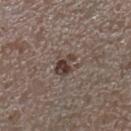follow-up: total-body-photography surveillance lesion; no biopsy
subject: female, about 70 years old
size: about 3.5 mm
lighting: white-light
anatomic site: the right lower leg
image: 15 mm crop, total-body photography
automated metrics: a mean CIELAB color near L≈39 a*≈14 b*≈19 and roughly 10 lightness units darker than nearby skin; an automated nevus-likeness rating near 75 out of 100 and a lesion-detection confidence of about 100/100